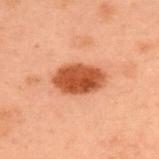Impression:
No biopsy was performed on this lesion — it was imaged during a full skin examination and was not determined to be concerning.
Context:
A male subject aged approximately 55. A 15 mm crop from a total-body photograph taken for skin-cancer surveillance. About 5.5 mm across. Located on the upper back.